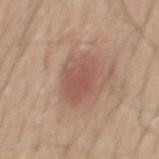Impression:
The lesion was tiled from a total-body skin photograph and was not biopsied.
Clinical summary:
A roughly 15 mm field-of-view crop from a total-body skin photograph. Located on the mid back. A male patient aged 63–67.